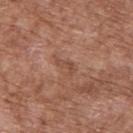follow-up — catalogued during a skin exam; not biopsied
anatomic site — the back
imaging modality — ~15 mm tile from a whole-body skin photo
subject — male, in their mid-70s